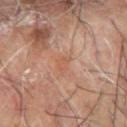tile lighting — cross-polarized illumination | TBP lesion metrics — a lesion area of about 2.5 mm², an eccentricity of roughly 0.85, and a shape-asymmetry score of about 0.4 (0 = symmetric); a border-irregularity index near 4/10, internal color variation of about 0 on a 0–10 scale, and peripheral color asymmetry of about 0 | body site — the left forearm | image — ~15 mm crop, total-body skin-cancer survey | patient — male, aged approximately 65 | lesion diameter — about 2.5 mm.